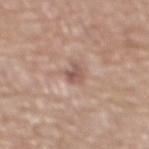Q: Is there a histopathology result?
A: no biopsy performed (imaged during a skin exam)
Q: Automated lesion metrics?
A: an eccentricity of roughly 0.75; a mean CIELAB color near L≈54 a*≈19 b*≈24, roughly 11 lightness units darker than nearby skin, and a normalized lesion–skin contrast near 7.5; a border-irregularity index near 3.5/10, internal color variation of about 1.5 on a 0–10 scale, and radial color variation of about 0.5; an automated nevus-likeness rating near 0 out of 100 and a detector confidence of about 100 out of 100 that the crop contains a lesion
Q: How was the tile lit?
A: white-light illumination
Q: Who is the patient?
A: male, in their 70s
Q: How was this image acquired?
A: 15 mm crop, total-body photography
Q: Lesion location?
A: the chest